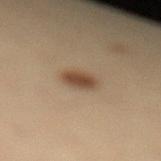Clinical impression: Imaged during a routine full-body skin examination; the lesion was not biopsied and no histopathology is available. Image and clinical context: The tile uses cross-polarized illumination. A 15 mm crop from a total-body photograph taken for skin-cancer surveillance. On the left lower leg. Approximately 2.5 mm at its widest. A female patient aged approximately 30.